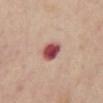No biopsy was performed on this lesion — it was imaged during a full skin examination and was not determined to be concerning.
Located on the chest.
Automated image analysis of the tile measured an average lesion color of about L≈50 a*≈31 b*≈22 (CIELAB), roughly 19 lightness units darker than nearby skin, and a normalized lesion–skin contrast near 13. The software also gave a border-irregularity index near 1.5/10, a color-variation rating of about 5/10, and a peripheral color-asymmetry measure near 1.5.
A male patient about 55 years old.
This is a white-light tile.
A 15 mm crop from a total-body photograph taken for skin-cancer surveillance.
Approximately 3 mm at its widest.